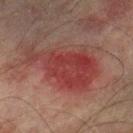  biopsy_status: not biopsied; imaged during a skin examination
  lesion_size:
    long_diameter_mm_approx: 8.5
  site: right lower leg
  lighting: cross-polarized
  image:
    source: total-body photography crop
    field_of_view_mm: 15
  patient:
    sex: male
    age_approx: 75